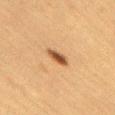follow-up: total-body-photography surveillance lesion; no biopsy | lesion size: ≈3 mm | anatomic site: the lower back | illumination: cross-polarized | patient: female, in their mid- to late 50s | image source: 15 mm crop, total-body photography.